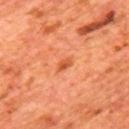{"biopsy_status": "not biopsied; imaged during a skin examination", "site": "mid back", "lighting": "cross-polarized", "patient": {"sex": "male", "age_approx": 65}, "image": {"source": "total-body photography crop", "field_of_view_mm": 15}, "lesion_size": {"long_diameter_mm_approx": 2.0}}